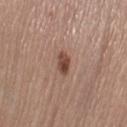Notes:
- follow-up: total-body-photography surveillance lesion; no biopsy
- imaging modality: 15 mm crop, total-body photography
- subject: female, approximately 65 years of age
- tile lighting: white-light
- anatomic site: the leg
- size: ≈3 mm